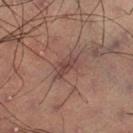notes=no biopsy performed (imaged during a skin exam); image=15 mm crop, total-body photography; patient=male, approximately 60 years of age; size=≈3.5 mm.